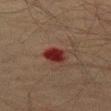<lesion>
  <biopsy_status>not biopsied; imaged during a skin examination</biopsy_status>
  <site>left thigh</site>
  <image>
    <source>total-body photography crop</source>
    <field_of_view_mm>15</field_of_view_mm>
  </image>
  <patient>
    <sex>male</sex>
    <age_approx>50</age_approx>
  </patient>
</lesion>